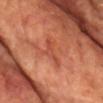| key | value |
|---|---|
| follow-up | total-body-photography surveillance lesion; no biopsy |
| TBP lesion metrics | a lesion area of about 4.5 mm², a shape eccentricity near 0.95, and a symmetry-axis asymmetry near 0.55; an average lesion color of about L≈51 a*≈33 b*≈35 (CIELAB), about 7 CIELAB-L* units darker than the surrounding skin, and a lesion-to-skin contrast of about 5 (normalized; higher = more distinct); a detector confidence of about 85 out of 100 that the crop contains a lesion |
| subject | male, aged 63 to 67 |
| location | the chest |
| image source | ~15 mm crop, total-body skin-cancer survey |